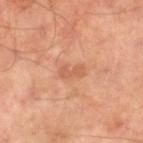{
  "biopsy_status": "not biopsied; imaged during a skin examination",
  "patient": {
    "sex": "male",
    "age_approx": 50
  },
  "lesion_size": {
    "long_diameter_mm_approx": 3.0
  },
  "lighting": "cross-polarized",
  "image": {
    "source": "total-body photography crop",
    "field_of_view_mm": 15
  },
  "site": "left leg"
}